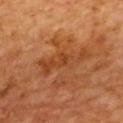Imaged during a routine full-body skin examination; the lesion was not biopsied and no histopathology is available.
The lesion is on the upper back.
A male subject aged around 65.
A close-up tile cropped from a whole-body skin photograph, about 15 mm across.
Measured at roughly 6.5 mm in maximum diameter.
Automated image analysis of the tile measured a footprint of about 11 mm², an outline eccentricity of about 0.95 (0 = round, 1 = elongated), and two-axis asymmetry of about 0.5. It also reported a nevus-likeness score of about 0/100.